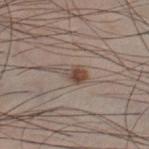* notes · catalogued during a skin exam; not biopsied